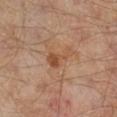Q: What is the lesion's diameter?
A: ≈3.5 mm
Q: What did automated image analysis measure?
A: a lesion area of about 5 mm², an outline eccentricity of about 0.85 (0 = round, 1 = elongated), and two-axis asymmetry of about 0.65; an average lesion color of about L≈49 a*≈21 b*≈33 (CIELAB), roughly 8 lightness units darker than nearby skin, and a lesion-to-skin contrast of about 6.5 (normalized; higher = more distinct); a color-variation rating of about 2/10 and peripheral color asymmetry of about 0.5
Q: How was the tile lit?
A: cross-polarized illumination
Q: How was this image acquired?
A: total-body-photography crop, ~15 mm field of view
Q: What are the patient's age and sex?
A: aged 53–57
Q: What is the anatomic site?
A: the right lower leg